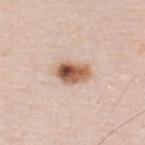| feature | finding |
|---|---|
| biopsy status | total-body-photography surveillance lesion; no biopsy |